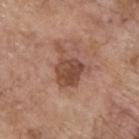Part of a total-body skin-imaging series; this lesion was reviewed on a skin check and was not flagged for biopsy. On the upper back. A lesion tile, about 15 mm wide, cut from a 3D total-body photograph. A male subject in their 70s.